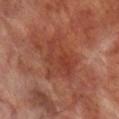Q: Is there a histopathology result?
A: catalogued during a skin exam; not biopsied
Q: Automated lesion metrics?
A: a lesion area of about 16 mm² and a shape-asymmetry score of about 0.45 (0 = symmetric); a classifier nevus-likeness of about 0/100 and a lesion-detection confidence of about 100/100
Q: What is the anatomic site?
A: the leg
Q: Patient demographics?
A: male, in their 70s
Q: What is the lesion's diameter?
A: ≈5.5 mm
Q: How was this image acquired?
A: ~15 mm crop, total-body skin-cancer survey